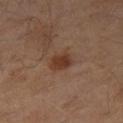Q: Was this lesion biopsied?
A: imaged on a skin check; not biopsied
Q: Who is the patient?
A: male, in their 60s
Q: What is the anatomic site?
A: the right thigh
Q: What lighting was used for the tile?
A: cross-polarized
Q: What kind of image is this?
A: ~15 mm crop, total-body skin-cancer survey